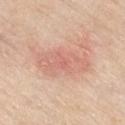Q: Was a biopsy performed?
A: catalogued during a skin exam; not biopsied
Q: How was this image acquired?
A: 15 mm crop, total-body photography
Q: What is the anatomic site?
A: the front of the torso
Q: Lesion size?
A: ≈6.5 mm
Q: What lighting was used for the tile?
A: white-light illumination
Q: Patient demographics?
A: female, aged 68–72